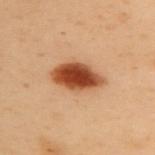The lesion was photographed on a routine skin check and not biopsied; there is no pathology result. The recorded lesion diameter is about 6 mm. Located on the upper back. This is a cross-polarized tile. A female subject aged approximately 60. Cropped from a whole-body photographic skin survey; the tile spans about 15 mm.